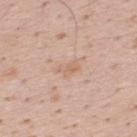Imaged during a routine full-body skin examination; the lesion was not biopsied and no histopathology is available.
The lesion is on the back.
A male subject about 35 years old.
Cropped from a whole-body photographic skin survey; the tile spans about 15 mm.
The recorded lesion diameter is about 2.5 mm.